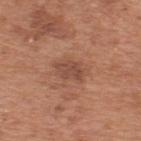biopsy status: no biopsy performed (imaged during a skin exam) | patient: male, roughly 65 years of age | automated lesion analysis: a lesion area of about 5.5 mm², a shape eccentricity near 0.8, and a symmetry-axis asymmetry near 0.3; border irregularity of about 3 on a 0–10 scale, a within-lesion color-variation index near 2/10, and a peripheral color-asymmetry measure near 1; a nevus-likeness score of about 15/100 and a lesion-detection confidence of about 100/100 | lesion diameter: ~3.5 mm (longest diameter) | image: 15 mm crop, total-body photography | site: the back.